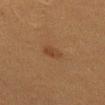Recorded during total-body skin imaging; not selected for excision or biopsy. Automated image analysis of the tile measured a classifier nevus-likeness of about 75/100. The lesion is on the left thigh. Imaged with cross-polarized lighting. A female patient, roughly 40 years of age. A close-up tile cropped from a whole-body skin photograph, about 15 mm across. Longest diameter approximately 2.5 mm.